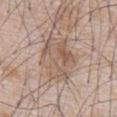This image is a 15 mm lesion crop taken from a total-body photograph. From the chest. The lesion-visualizer software estimated an area of roughly 18 mm², an outline eccentricity of about 0.8 (0 = round, 1 = elongated), and two-axis asymmetry of about 0.35. The analysis additionally found a lesion color around L≈57 a*≈15 b*≈26 in CIELAB and a normalized border contrast of about 6. It also reported a detector confidence of about 85 out of 100 that the crop contains a lesion. A male patient aged around 65. This is a white-light tile. The recorded lesion diameter is about 6.5 mm.